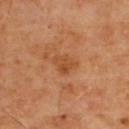follow-up = no biopsy performed (imaged during a skin exam)
anatomic site = the upper back
patient = male, roughly 65 years of age
imaging modality = ~15 mm crop, total-body skin-cancer survey
lighting = cross-polarized illumination
diameter = ~3 mm (longest diameter)